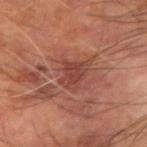Q: Is there a histopathology result?
A: total-body-photography surveillance lesion; no biopsy
Q: How was this image acquired?
A: ~15 mm crop, total-body skin-cancer survey
Q: Lesion size?
A: about 3 mm
Q: What are the patient's age and sex?
A: male, roughly 60 years of age
Q: What is the anatomic site?
A: the arm